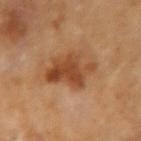Assessment: Part of a total-body skin-imaging series; this lesion was reviewed on a skin check and was not flagged for biopsy. Acquisition and patient details: On the left forearm. A female subject, aged 58–62. A close-up tile cropped from a whole-body skin photograph, about 15 mm across. Approximately 6 mm at its widest. Captured under cross-polarized illumination. The total-body-photography lesion software estimated an outline eccentricity of about 0.85 (0 = round, 1 = elongated) and a shape-asymmetry score of about 0.4 (0 = symmetric). It also reported border irregularity of about 5 on a 0–10 scale. The analysis additionally found an automated nevus-likeness rating near 55 out of 100 and lesion-presence confidence of about 100/100.